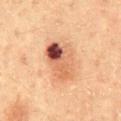  biopsy_status: not biopsied; imaged during a skin examination
  image:
    source: total-body photography crop
    field_of_view_mm: 15
  site: abdomen
  patient:
    sex: male
    age_approx: 60